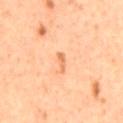  biopsy_status: not biopsied; imaged during a skin examination
  patient:
    sex: male
    age_approx: 60
  image:
    source: total-body photography crop
    field_of_view_mm: 15
  site: mid back